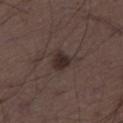| feature | finding |
|---|---|
| image | 15 mm crop, total-body photography |
| anatomic site | the right thigh |
| patient | male, approximately 50 years of age |